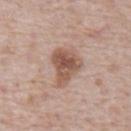This lesion was catalogued during total-body skin photography and was not selected for biopsy. A male patient roughly 70 years of age. A roughly 15 mm field-of-view crop from a total-body skin photograph. Located on the abdomen.